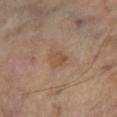<record>
<biopsy_status>not biopsied; imaged during a skin examination</biopsy_status>
<patient>
  <age_approx>55</age_approx>
</patient>
<site>left lower leg</site>
<image>
  <source>total-body photography crop</source>
  <field_of_view_mm>15</field_of_view_mm>
</image>
<lesion_size>
  <long_diameter_mm_approx>2.5</long_diameter_mm_approx>
</lesion_size>
</record>